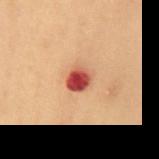Part of a total-body skin-imaging series; this lesion was reviewed on a skin check and was not flagged for biopsy. Longest diameter approximately 3 mm. The tile uses cross-polarized illumination. The lesion is on the mid back. A roughly 15 mm field-of-view crop from a total-body skin photograph. The patient is a male roughly 55 years of age.